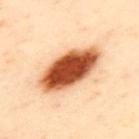Q: Was a biopsy performed?
A: total-body-photography surveillance lesion; no biopsy
Q: What is the lesion's diameter?
A: about 8 mm
Q: Patient demographics?
A: female, aged 38–42
Q: What kind of image is this?
A: ~15 mm crop, total-body skin-cancer survey
Q: What is the anatomic site?
A: the upper back
Q: Illumination type?
A: cross-polarized